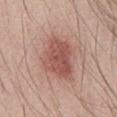* notes — no biopsy performed (imaged during a skin exam)
* lighting — white-light illumination
* acquisition — ~15 mm crop, total-body skin-cancer survey
* patient — male, aged around 25
* anatomic site — the chest